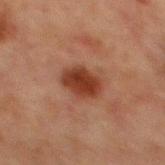workup = total-body-photography surveillance lesion; no biopsy | subject = male, approximately 65 years of age | site = the chest | acquisition = ~15 mm crop, total-body skin-cancer survey.